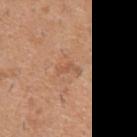Imaged during a routine full-body skin examination; the lesion was not biopsied and no histopathology is available. This is a white-light tile. Automated tile analysis of the lesion measured a border-irregularity index near 6.5/10 and radial color variation of about 0. It also reported a nevus-likeness score of about 0/100. A 15 mm close-up tile from a total-body photography series done for melanoma screening. The lesion is on the left upper arm. The patient is a male aged 38–42. The recorded lesion diameter is about 3 mm.